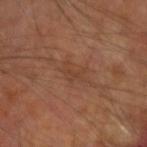A male patient, aged around 65. A 15 mm close-up tile from a total-body photography series done for melanoma screening. The recorded lesion diameter is about 3 mm. On the left arm. Automated tile analysis of the lesion measured an eccentricity of roughly 0.8 and a symmetry-axis asymmetry near 0.6. And it measured border irregularity of about 6.5 on a 0–10 scale and internal color variation of about 0 on a 0–10 scale. The analysis additionally found a lesion-detection confidence of about 90/100. Imaged with cross-polarized lighting.